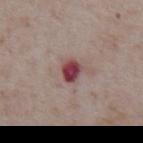  biopsy_status: not biopsied; imaged during a skin examination
  site: abdomen
  automated_metrics:
    eccentricity: 0.6
    shape_asymmetry: 0.2
    nevus_likeness_0_100: 0
  lesion_size:
    long_diameter_mm_approx: 2.5
  image:
    source: total-body photography crop
    field_of_view_mm: 15
  patient:
    sex: male
    age_approx: 75